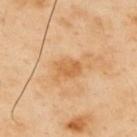Q: Was this lesion biopsied?
A: no biopsy performed (imaged during a skin exam)
Q: What lighting was used for the tile?
A: cross-polarized
Q: What kind of image is this?
A: ~15 mm crop, total-body skin-cancer survey
Q: What is the anatomic site?
A: the upper back
Q: How large is the lesion?
A: ≈3 mm
Q: Automated lesion metrics?
A: a lesion area of about 5.5 mm², an eccentricity of roughly 0.7, and two-axis asymmetry of about 0.2; a lesion color around L≈63 a*≈22 b*≈43 in CIELAB, about 8 CIELAB-L* units darker than the surrounding skin, and a normalized lesion–skin contrast near 6
Q: What are the patient's age and sex?
A: male, roughly 55 years of age